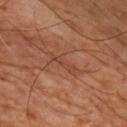<record>
<biopsy_status>not biopsied; imaged during a skin examination</biopsy_status>
<site>chest</site>
<lesion_size>
  <long_diameter_mm_approx>4.5</long_diameter_mm_approx>
</lesion_size>
<image>
  <source>total-body photography crop</source>
  <field_of_view_mm>15</field_of_view_mm>
</image>
<automated_metrics>
  <border_irregularity_0_10>5.5</border_irregularity_0_10>
  <peripheral_color_asymmetry>0.0</peripheral_color_asymmetry>
</automated_metrics>
<lighting>cross-polarized</lighting>
<patient>
  <sex>male</sex>
  <age_approx>55</age_approx>
</patient>
</record>